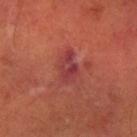Notes:
– biopsy status: imaged on a skin check; not biopsied
– patient: male, aged 63–67
– diameter: about 3.5 mm
– illumination: cross-polarized illumination
– site: the arm
– image source: 15 mm crop, total-body photography